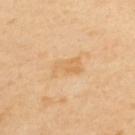tile lighting: cross-polarized illumination | anatomic site: the upper back | subject: male, aged approximately 55 | lesion size: about 3.5 mm | imaging modality: ~15 mm crop, total-body skin-cancer survey.